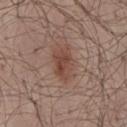| key | value |
|---|---|
| notes | imaged on a skin check; not biopsied |
| diameter | ≈4 mm |
| illumination | white-light |
| body site | the mid back |
| patient | male, roughly 50 years of age |
| automated metrics | an area of roughly 7.5 mm², an outline eccentricity of about 0.8 (0 = round, 1 = elongated), and a shape-asymmetry score of about 0.25 (0 = symmetric); a border-irregularity index near 2.5/10, a color-variation rating of about 3.5/10, and a peripheral color-asymmetry measure near 1; a lesion-detection confidence of about 100/100 |
| acquisition | total-body-photography crop, ~15 mm field of view |